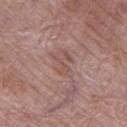No biopsy was performed on this lesion — it was imaged during a full skin examination and was not determined to be concerning. A female subject, aged around 70. The lesion's longest dimension is about 3.5 mm. On the left thigh. Captured under white-light illumination. Cropped from a total-body skin-imaging series; the visible field is about 15 mm.